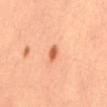| key | value |
|---|---|
| workup | imaged on a skin check; not biopsied |
| anatomic site | the back |
| patient | male, about 35 years old |
| image | 15 mm crop, total-body photography |